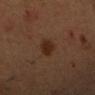Clinical impression: Imaged during a routine full-body skin examination; the lesion was not biopsied and no histopathology is available. Clinical summary: This is a cross-polarized tile. Measured at roughly 3 mm in maximum diameter. The subject is a male aged 33 to 37. Cropped from a whole-body photographic skin survey; the tile spans about 15 mm. The lesion is on the head or neck.